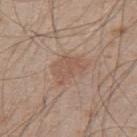workup: total-body-photography surveillance lesion; no biopsy | tile lighting: white-light illumination | body site: the upper back | subject: male, about 50 years old | image source: ~15 mm tile from a whole-body skin photo | diameter: ~4.5 mm (longest diameter).